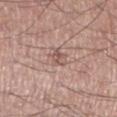The lesion was photographed on a routine skin check and not biopsied; there is no pathology result. A male patient, aged around 45. An algorithmic analysis of the crop reported an outline eccentricity of about 0.85 (0 = round, 1 = elongated) and a shape-asymmetry score of about 0.3 (0 = symmetric). It also reported an average lesion color of about L≈55 a*≈19 b*≈24 (CIELAB), a lesion–skin lightness drop of about 9, and a lesion-to-skin contrast of about 6 (normalized; higher = more distinct). The analysis additionally found a border-irregularity rating of about 3.5/10 and peripheral color asymmetry of about 1. The lesion's longest dimension is about 3 mm. This image is a 15 mm lesion crop taken from a total-body photograph. This is a white-light tile. The lesion is on the right lower leg.